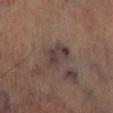Imaged during a routine full-body skin examination; the lesion was not biopsied and no histopathology is available.
The lesion's longest dimension is about 4 mm.
Located on the right lower leg.
This is a cross-polarized tile.
This image is a 15 mm lesion crop taken from a total-body photograph.